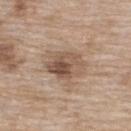Context:
A female patient, roughly 75 years of age. Measured at roughly 5 mm in maximum diameter. The tile uses white-light illumination. Cropped from a total-body skin-imaging series; the visible field is about 15 mm. Located on the upper back.